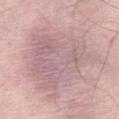Part of a total-body skin-imaging series; this lesion was reviewed on a skin check and was not flagged for biopsy.
A 15 mm close-up extracted from a 3D total-body photography capture.
The lesion-visualizer software estimated a mean CIELAB color near L≈65 a*≈19 b*≈18, a lesion–skin lightness drop of about 8, and a normalized lesion–skin contrast near 5. It also reported a border-irregularity rating of about 5.5/10, a within-lesion color-variation index near 4.5/10, and peripheral color asymmetry of about 1.5. The software also gave a nevus-likeness score of about 0/100.
A female subject in their mid- to late 60s.
On the abdomen.